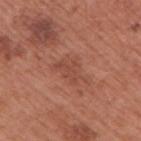The lesion was tiled from a total-body skin photograph and was not biopsied. Captured under white-light illumination. On the right upper arm. Automated tile analysis of the lesion measured a lesion-to-skin contrast of about 5 (normalized; higher = more distinct). The software also gave a nevus-likeness score of about 0/100 and a lesion-detection confidence of about 100/100. Cropped from a whole-body photographic skin survey; the tile spans about 15 mm. A male patient, approximately 70 years of age.